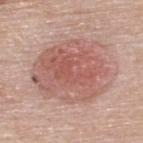Assessment:
The lesion was photographed on a routine skin check and not biopsied; there is no pathology result.
Context:
From the upper back. Automated tile analysis of the lesion measured a lesion color around L≈58 a*≈23 b*≈25 in CIELAB and a lesion-to-skin contrast of about 7 (normalized; higher = more distinct). The software also gave an automated nevus-likeness rating near 95 out of 100 and a lesion-detection confidence of about 100/100. Measured at roughly 8.5 mm in maximum diameter. A female subject, roughly 50 years of age. Imaged with white-light lighting. A region of skin cropped from a whole-body photographic capture, roughly 15 mm wide.